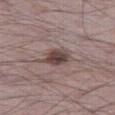notes — total-body-photography surveillance lesion; no biopsy | automated lesion analysis — a footprint of about 7.5 mm² and an outline eccentricity of about 0.5 (0 = round, 1 = elongated); a lesion color around L≈44 a*≈15 b*≈18 in CIELAB, about 12 CIELAB-L* units darker than the surrounding skin, and a normalized lesion–skin contrast near 9; a color-variation rating of about 4.5/10 and a peripheral color-asymmetry measure near 1.5 | tile lighting — white-light | subject — male, aged 68–72 | imaging modality — total-body-photography crop, ~15 mm field of view | location — the leg | lesion diameter — about 3.5 mm.